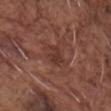Case summary:
– notes — imaged on a skin check; not biopsied
– anatomic site — the front of the torso
– subject — male, aged 73–77
– imaging modality — total-body-photography crop, ~15 mm field of view